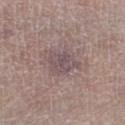Clinical impression: Captured during whole-body skin photography for melanoma surveillance; the lesion was not biopsied. Background: Automated tile analysis of the lesion measured a footprint of about 7.5 mm² and a symmetry-axis asymmetry near 0.3. It also reported a lesion color around L≈49 a*≈15 b*≈14 in CIELAB, a lesion–skin lightness drop of about 8, and a normalized lesion–skin contrast near 7. And it measured border irregularity of about 3.5 on a 0–10 scale and a peripheral color-asymmetry measure near 0.5. This is a white-light tile. A region of skin cropped from a whole-body photographic capture, roughly 15 mm wide. Located on the right thigh. About 3.5 mm across. A male patient, aged approximately 80.